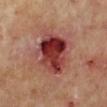Imaged during a routine full-body skin examination; the lesion was not biopsied and no histopathology is available. A 15 mm close-up extracted from a 3D total-body photography capture. The patient is a male aged 63 to 67. The lesion-visualizer software estimated an area of roughly 21 mm², an outline eccentricity of about 0.45 (0 = round, 1 = elongated), and a shape-asymmetry score of about 0.15 (0 = symmetric). From the left lower leg.